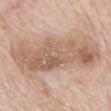Clinical impression:
Recorded during total-body skin imaging; not selected for excision or biopsy.
Clinical summary:
A male patient approximately 85 years of age or older. Measured at roughly 13 mm in maximum diameter. A 15 mm crop from a total-body photograph taken for skin-cancer surveillance. The tile uses white-light illumination. From the mid back.